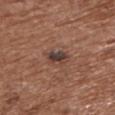The lesion was photographed on a routine skin check and not biopsied; there is no pathology result. On the chest. Approximately 3 mm at its widest. A roughly 15 mm field-of-view crop from a total-body skin photograph. The subject is a female aged 73–77. Captured under white-light illumination.